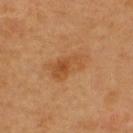Findings:
- anatomic site: the upper back
- illumination: cross-polarized illumination
- lesion diameter: ≈4.5 mm
- image: ~15 mm tile from a whole-body skin photo
- subject: male, roughly 45 years of age